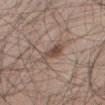Recorded during total-body skin imaging; not selected for excision or biopsy.
Imaged with white-light lighting.
A close-up tile cropped from a whole-body skin photograph, about 15 mm across.
Located on the right thigh.
A male subject aged 43 to 47.
The lesion's longest dimension is about 4 mm.
An algorithmic analysis of the crop reported a border-irregularity rating of about 3.5/10, a within-lesion color-variation index near 3/10, and radial color variation of about 0.5.